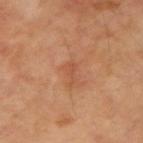The lesion was tiled from a total-body skin photograph and was not biopsied.
On the right upper arm.
A male patient, approximately 70 years of age.
The total-body-photography lesion software estimated a border-irregularity rating of about 6/10, a color-variation rating of about 0/10, and a peripheral color-asymmetry measure near 0. It also reported a nevus-likeness score of about 0/100.
Approximately 2.5 mm at its widest.
Captured under cross-polarized illumination.
A 15 mm close-up extracted from a 3D total-body photography capture.